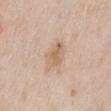The lesion was photographed on a routine skin check and not biopsied; there is no pathology result. Automated tile analysis of the lesion measured a footprint of about 6 mm² and a shape eccentricity near 0.8. It also reported a mean CIELAB color near L≈63 a*≈18 b*≈31, roughly 8 lightness units darker than nearby skin, and a normalized border contrast of about 6. A male subject, in their mid- to late 60s. This is a white-light tile. The lesion's longest dimension is about 3.5 mm. On the abdomen. A lesion tile, about 15 mm wide, cut from a 3D total-body photograph.